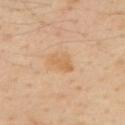Q: Was a biopsy performed?
A: catalogued during a skin exam; not biopsied
Q: Where on the body is the lesion?
A: the mid back
Q: What kind of image is this?
A: total-body-photography crop, ~15 mm field of view
Q: What are the patient's age and sex?
A: male, about 50 years old